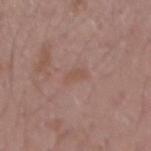follow-up: catalogued during a skin exam; not biopsied | body site: the arm | lesion diameter: ≈2.5 mm | patient: male, in their 20s | acquisition: ~15 mm tile from a whole-body skin photo | illumination: white-light illumination.